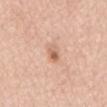Findings:
• size · ~2.5 mm (longest diameter)
• anatomic site · the abdomen
• imaging modality · ~15 mm crop, total-body skin-cancer survey
• image-analysis metrics · a lesion–skin lightness drop of about 11 and a normalized lesion–skin contrast near 7
• patient · male, aged around 75
• lighting · white-light